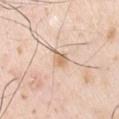This lesion was catalogued during total-body skin photography and was not selected for biopsy. A region of skin cropped from a whole-body photographic capture, roughly 15 mm wide. The tile uses white-light illumination. The lesion is located on the chest. The total-body-photography lesion software estimated an eccentricity of roughly 0.7. The software also gave an average lesion color of about L≈69 a*≈16 b*≈33 (CIELAB), a lesion–skin lightness drop of about 10, and a normalized lesion–skin contrast near 6.5. A male patient in their 40s. Approximately 2.5 mm at its widest.